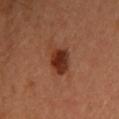Clinical summary: A female patient roughly 35 years of age. Measured at roughly 4 mm in maximum diameter. A 15 mm close-up extracted from a 3D total-body photography capture. On the head or neck. An algorithmic analysis of the crop reported an eccentricity of roughly 0.75. And it measured a border-irregularity rating of about 3/10, a within-lesion color-variation index near 5/10, and peripheral color asymmetry of about 1.5. The tile uses cross-polarized illumination.